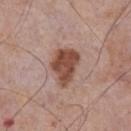Impression:
Part of a total-body skin-imaging series; this lesion was reviewed on a skin check and was not flagged for biopsy.
Context:
The lesion's longest dimension is about 5 mm. The lesion is located on the chest. The patient is a male aged 73–77. The tile uses white-light illumination. A close-up tile cropped from a whole-body skin photograph, about 15 mm across.